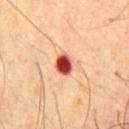Clinical impression: This lesion was catalogued during total-body skin photography and was not selected for biopsy. Context: Approximately 2.5 mm at its widest. A male patient roughly 65 years of age. From the upper back. Automated tile analysis of the lesion measured a mean CIELAB color near L≈47 a*≈38 b*≈32, roughly 23 lightness units darker than nearby skin, and a normalized border contrast of about 15. The software also gave a classifier nevus-likeness of about 0/100 and a lesion-detection confidence of about 100/100. A 15 mm close-up tile from a total-body photography series done for melanoma screening. The tile uses cross-polarized illumination.